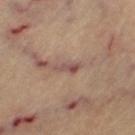Clinical impression:
Imaged during a routine full-body skin examination; the lesion was not biopsied and no histopathology is available.
Background:
Automated image analysis of the tile measured an outline eccentricity of about 0.9 (0 = round, 1 = elongated) and a symmetry-axis asymmetry near 0.45. The analysis additionally found a detector confidence of about 60 out of 100 that the crop contains a lesion. The tile uses cross-polarized illumination. The lesion's longest dimension is about 3 mm. A female patient, aged 63 to 67. A roughly 15 mm field-of-view crop from a total-body skin photograph. The lesion is located on the left thigh.